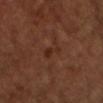follow-up = no biopsy performed (imaged during a skin exam), location = the head or neck, acquisition = ~15 mm tile from a whole-body skin photo.